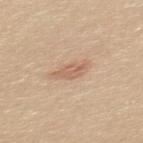Q: Was a biopsy performed?
A: catalogued during a skin exam; not biopsied
Q: How was the tile lit?
A: white-light
Q: Who is the patient?
A: female, aged around 20
Q: What did automated image analysis measure?
A: an area of roughly 4.5 mm², an outline eccentricity of about 0.85 (0 = round, 1 = elongated), and a shape-asymmetry score of about 0.25 (0 = symmetric); an average lesion color of about L≈62 a*≈20 b*≈31 (CIELAB) and a lesion–skin lightness drop of about 9
Q: Where on the body is the lesion?
A: the upper back
Q: How was this image acquired?
A: total-body-photography crop, ~15 mm field of view